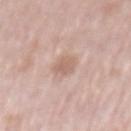Q: Is there a histopathology result?
A: no biopsy performed (imaged during a skin exam)
Q: Who is the patient?
A: male, about 75 years old
Q: How was this image acquired?
A: ~15 mm tile from a whole-body skin photo
Q: How was the tile lit?
A: white-light illumination
Q: Lesion size?
A: ≈3 mm
Q: What is the anatomic site?
A: the mid back
Q: What did automated image analysis measure?
A: an automated nevus-likeness rating near 40 out of 100 and lesion-presence confidence of about 100/100